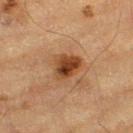Notes:
- follow-up · no biopsy performed (imaged during a skin exam)
- location · the left thigh
- lesion diameter · ≈3 mm
- patient · male, aged approximately 85 or older
- imaging modality · 15 mm crop, total-body photography
- tile lighting · cross-polarized